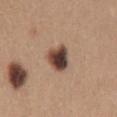Impression: No biopsy was performed on this lesion — it was imaged during a full skin examination and was not determined to be concerning. Image and clinical context: The recorded lesion diameter is about 3.5 mm. The lesion-visualizer software estimated a mean CIELAB color near L≈42 a*≈18 b*≈25 and a normalized lesion–skin contrast near 14.5. It also reported a within-lesion color-variation index near 8.5/10 and peripheral color asymmetry of about 2. The analysis additionally found a nevus-likeness score of about 95/100. The lesion is located on the abdomen. A female patient in their mid- to late 40s. A lesion tile, about 15 mm wide, cut from a 3D total-body photograph.